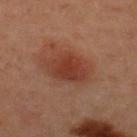A 15 mm close-up tile from a total-body photography series done for melanoma screening. A female patient aged 43 to 47. The lesion is located on the upper back.